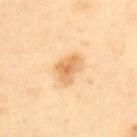Q: How was this image acquired?
A: ~15 mm crop, total-body skin-cancer survey
Q: What is the anatomic site?
A: the back
Q: Patient demographics?
A: male, aged around 65